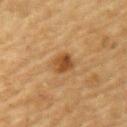Assessment: The lesion was photographed on a routine skin check and not biopsied; there is no pathology result. Clinical summary: The patient is a male about 85 years old or older. A 15 mm close-up tile from a total-body photography series done for melanoma screening. About 3 mm across. The lesion is on the upper back. Captured under cross-polarized illumination.